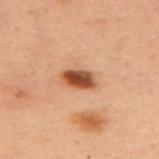Part of a total-body skin-imaging series; this lesion was reviewed on a skin check and was not flagged for biopsy.